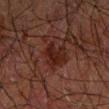Notes:
– workup: catalogued during a skin exam; not biopsied
– diameter: ≈3.5 mm
– body site: the right forearm
– patient: male, approximately 70 years of age
– automated metrics: an average lesion color of about L≈17 a*≈20 b*≈20 (CIELAB), a lesion–skin lightness drop of about 7, and a lesion-to-skin contrast of about 9 (normalized; higher = more distinct); a nevus-likeness score of about 0/100
– lighting: cross-polarized
– imaging modality: ~15 mm crop, total-body skin-cancer survey